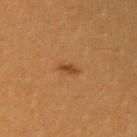No biopsy was performed on this lesion — it was imaged during a full skin examination and was not determined to be concerning. Automated image analysis of the tile measured a footprint of about 1.5 mm², an outline eccentricity of about 0.9 (0 = round, 1 = elongated), and a symmetry-axis asymmetry near 0.3. And it measured a color-variation rating of about 0/10. And it measured a detector confidence of about 100 out of 100 that the crop contains a lesion. A female patient, approximately 30 years of age. This is a cross-polarized tile. Approximately 2 mm at its widest. Cropped from a total-body skin-imaging series; the visible field is about 15 mm. The lesion is located on the arm.